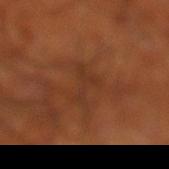biopsy status: total-body-photography surveillance lesion; no biopsy
acquisition: total-body-photography crop, ~15 mm field of view
body site: the leg
image-analysis metrics: a lesion–skin lightness drop of about 5; internal color variation of about 0 on a 0–10 scale and peripheral color asymmetry of about 0
tile lighting: cross-polarized
diameter: ≈4 mm
patient: male, aged approximately 70